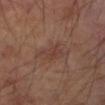Case summary:
– workup — imaged on a skin check; not biopsied